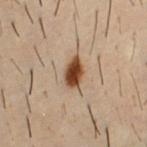Captured during whole-body skin photography for melanoma surveillance; the lesion was not biopsied.
About 3.5 mm across.
A close-up tile cropped from a whole-body skin photograph, about 15 mm across.
Captured under cross-polarized illumination.
From the front of the torso.
A male patient, aged around 30.
The lesion-visualizer software estimated a lesion color around L≈36 a*≈17 b*≈28 in CIELAB, roughly 15 lightness units darker than nearby skin, and a normalized lesion–skin contrast near 13.5. It also reported a color-variation rating of about 3/10 and a peripheral color-asymmetry measure near 1.